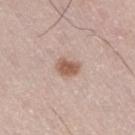notes=no biopsy performed (imaged during a skin exam); patient=male, in their mid-60s; diameter=~3 mm (longest diameter); image source=~15 mm tile from a whole-body skin photo; site=the right thigh.